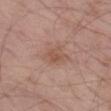No biopsy was performed on this lesion — it was imaged during a full skin examination and was not determined to be concerning.
The lesion is on the left thigh.
A region of skin cropped from a whole-body photographic capture, roughly 15 mm wide.
A male patient aged around 55.